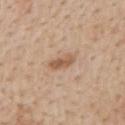follow-up=no biopsy performed (imaged during a skin exam)
body site=the mid back
illumination=white-light illumination
patient=male, aged 58–62
lesion diameter=about 3.5 mm
image=~15 mm tile from a whole-body skin photo